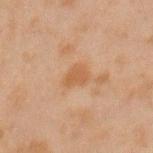The lesion was tiled from a total-body skin photograph and was not biopsied. A male subject, aged approximately 45. The lesion-visualizer software estimated a lesion area of about 4.5 mm², an outline eccentricity of about 0.65 (0 = round, 1 = elongated), and two-axis asymmetry of about 0.3. It also reported a lesion color around L≈46 a*≈18 b*≈31 in CIELAB, about 6 CIELAB-L* units darker than the surrounding skin, and a lesion-to-skin contrast of about 6.5 (normalized; higher = more distinct). The software also gave a classifier nevus-likeness of about 0/100 and a detector confidence of about 100 out of 100 that the crop contains a lesion. Captured under cross-polarized illumination. A 15 mm close-up extracted from a 3D total-body photography capture. On the left forearm.